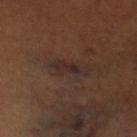Impression: This lesion was catalogued during total-body skin photography and was not selected for biopsy. Acquisition and patient details: A 15 mm close-up tile from a total-body photography series done for melanoma screening. The lesion's longest dimension is about 4 mm. The subject is a male aged 53 to 57. Automated image analysis of the tile measured an automated nevus-likeness rating near 50 out of 100 and lesion-presence confidence of about 75/100. Captured under cross-polarized illumination. The lesion is on the left lower leg.